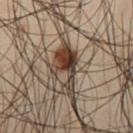patient: male, aged approximately 50
image: ~15 mm crop, total-body skin-cancer survey
size: ~6 mm (longest diameter)
TBP lesion metrics: an outline eccentricity of about 0.9 (0 = round, 1 = elongated) and a shape-asymmetry score of about 0.55 (0 = symmetric); about 12 CIELAB-L* units darker than the surrounding skin and a normalized border contrast of about 12; a nevus-likeness score of about 100/100 and lesion-presence confidence of about 90/100
site: the left thigh